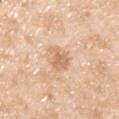No biopsy was performed on this lesion — it was imaged during a full skin examination and was not determined to be concerning.
A male patient aged approximately 45.
From the chest.
Automated image analysis of the tile measured a footprint of about 5.5 mm², an eccentricity of roughly 0.3, and a shape-asymmetry score of about 0.35 (0 = symmetric). It also reported a lesion color around L≈67 a*≈18 b*≈35 in CIELAB, a lesion–skin lightness drop of about 10, and a lesion-to-skin contrast of about 6.5 (normalized; higher = more distinct). The analysis additionally found a classifier nevus-likeness of about 0/100 and a detector confidence of about 100 out of 100 that the crop contains a lesion.
A close-up tile cropped from a whole-body skin photograph, about 15 mm across.
Measured at roughly 3 mm in maximum diameter.
This is a white-light tile.